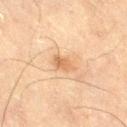Assessment: The lesion was photographed on a routine skin check and not biopsied; there is no pathology result. Context: From the leg. This is a cross-polarized tile. A male patient aged approximately 70. The lesion's longest dimension is about 2.5 mm. This image is a 15 mm lesion crop taken from a total-body photograph. Automated tile analysis of the lesion measured an average lesion color of about L≈55 a*≈18 b*≈33 (CIELAB), a lesion–skin lightness drop of about 8, and a normalized lesion–skin contrast near 6.